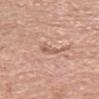<case>
  <lighting>white-light</lighting>
  <lesion_size>
    <long_diameter_mm_approx>2.5</long_diameter_mm_approx>
  </lesion_size>
  <image>
    <source>total-body photography crop</source>
    <field_of_view_mm>15</field_of_view_mm>
  </image>
  <patient>
    <sex>female</sex>
    <age_approx>65</age_approx>
  </patient>
  <site>head or neck</site>
</case>